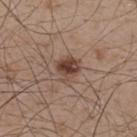biopsy status = no biopsy performed (imaged during a skin exam) | size = ≈3 mm | anatomic site = the upper back | subject = male, about 50 years old | acquisition = ~15 mm crop, total-body skin-cancer survey.